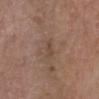Captured during whole-body skin photography for melanoma surveillance; the lesion was not biopsied. On the left forearm. This is a cross-polarized tile. The patient is a female approximately 70 years of age. A roughly 15 mm field-of-view crop from a total-body skin photograph.